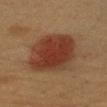follow-up: total-body-photography surveillance lesion; no biopsy | diameter: ~6 mm (longest diameter) | site: the arm | image-analysis metrics: a mean CIELAB color near L≈32 a*≈20 b*≈27, roughly 10 lightness units darker than nearby skin, and a normalized border contrast of about 10; a border-irregularity rating of about 1.5/10, internal color variation of about 4 on a 0–10 scale, and peripheral color asymmetry of about 1 | patient: female, about 40 years old | lighting: cross-polarized | imaging modality: 15 mm crop, total-body photography.